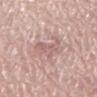follow-up=catalogued during a skin exam; not biopsied
image source=~15 mm crop, total-body skin-cancer survey
anatomic site=the back
subject=male, aged 78–82